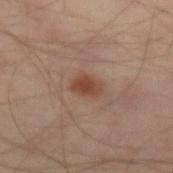Q: Was this lesion biopsied?
A: no biopsy performed (imaged during a skin exam)
Q: What are the patient's age and sex?
A: male, in their 50s
Q: What is the imaging modality?
A: ~15 mm crop, total-body skin-cancer survey
Q: How large is the lesion?
A: ~3 mm (longest diameter)
Q: What did automated image analysis measure?
A: a nevus-likeness score of about 95/100 and lesion-presence confidence of about 100/100
Q: How was the tile lit?
A: cross-polarized
Q: What is the anatomic site?
A: the left forearm